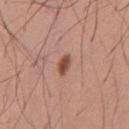Clinical impression: Recorded during total-body skin imaging; not selected for excision or biopsy. Background: Located on the chest. A male patient roughly 30 years of age. The lesion's longest dimension is about 3 mm. Captured under white-light illumination. This image is a 15 mm lesion crop taken from a total-body photograph.